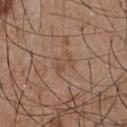| feature | finding |
|---|---|
| biopsy status | imaged on a skin check; not biopsied |
| patient | male, in their mid- to late 50s |
| lighting | white-light |
| site | the chest |
| imaging modality | ~15 mm crop, total-body skin-cancer survey |